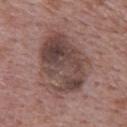Assessment: Part of a total-body skin-imaging series; this lesion was reviewed on a skin check and was not flagged for biopsy. Clinical summary: Approximately 8.5 mm at its widest. The subject is a male roughly 70 years of age. This is a white-light tile. The total-body-photography lesion software estimated internal color variation of about 7 on a 0–10 scale and peripheral color asymmetry of about 2.5. And it measured an automated nevus-likeness rating near 0 out of 100 and lesion-presence confidence of about 100/100. A 15 mm crop from a total-body photograph taken for skin-cancer surveillance. On the upper back.